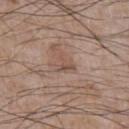biopsy status: imaged on a skin check; not biopsied
illumination: white-light
image source: total-body-photography crop, ~15 mm field of view
subject: male, aged around 75
lesion diameter: ~2.5 mm (longest diameter)
anatomic site: the chest